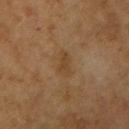A female subject about 60 years old.
A roughly 15 mm field-of-view crop from a total-body skin photograph.
The lesion is on the right upper arm.
Captured under cross-polarized illumination.